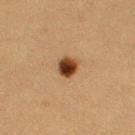notes=imaged on a skin check; not biopsied
image source=~15 mm crop, total-body skin-cancer survey
subject=female, approximately 40 years of age
anatomic site=the left upper arm